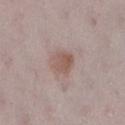The lesion was photographed on a routine skin check and not biopsied; there is no pathology result.
The lesion is located on the right lower leg.
A close-up tile cropped from a whole-body skin photograph, about 15 mm across.
This is a white-light tile.
The total-body-photography lesion software estimated a footprint of about 6.5 mm², a shape eccentricity near 0.5, and a symmetry-axis asymmetry near 0.25. And it measured about 9 CIELAB-L* units darker than the surrounding skin and a normalized border contrast of about 7. And it measured internal color variation of about 3.5 on a 0–10 scale and radial color variation of about 1.5. The software also gave a nevus-likeness score of about 75/100 and a lesion-detection confidence of about 100/100.
A female subject aged around 30.
Measured at roughly 3 mm in maximum diameter.